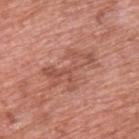workup: catalogued during a skin exam; not biopsied | imaging modality: ~15 mm tile from a whole-body skin photo | site: the upper back | automated lesion analysis: an average lesion color of about L≈53 a*≈25 b*≈29 (CIELAB), about 8 CIELAB-L* units darker than the surrounding skin, and a lesion-to-skin contrast of about 5.5 (normalized; higher = more distinct); a border-irregularity index near 10/10, a color-variation rating of about 4.5/10, and radial color variation of about 1.5 | lesion size: ~6.5 mm (longest diameter) | patient: male, aged around 70 | lighting: white-light illumination.